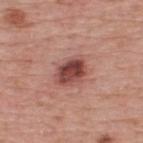follow-up = no biopsy performed (imaged during a skin exam)
acquisition = total-body-photography crop, ~15 mm field of view
lesion size = ~4 mm (longest diameter)
patient = male, aged 73 to 77
lighting = white-light illumination
anatomic site = the upper back
automated metrics = a border-irregularity rating of about 2/10, internal color variation of about 6 on a 0–10 scale, and radial color variation of about 2